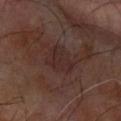{
  "biopsy_status": "not biopsied; imaged during a skin examination",
  "lighting": "cross-polarized",
  "patient": {
    "sex": "male",
    "age_approx": 65
  },
  "image": {
    "source": "total-body photography crop",
    "field_of_view_mm": 15
  },
  "site": "left forearm",
  "automated_metrics": {
    "area_mm2_approx": 9.0,
    "eccentricity": 0.7,
    "shape_asymmetry": 0.35,
    "color_variation_0_10": 2.5,
    "peripheral_color_asymmetry": 1.0,
    "nevus_likeness_0_100": 0,
    "lesion_detection_confidence_0_100": 100
  },
  "lesion_size": {
    "long_diameter_mm_approx": 4.0
  }
}